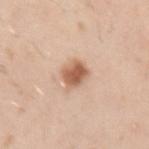Q: Was a biopsy performed?
A: catalogued during a skin exam; not biopsied
Q: How was the tile lit?
A: white-light
Q: Lesion location?
A: the left upper arm
Q: Automated lesion metrics?
A: an area of roughly 6.5 mm² and a shape-asymmetry score of about 0.2 (0 = symmetric); a mean CIELAB color near L≈60 a*≈22 b*≈32 and a normalized lesion–skin contrast near 9.5
Q: What are the patient's age and sex?
A: male, aged around 30
Q: What is the imaging modality?
A: 15 mm crop, total-body photography